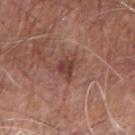Clinical impression:
Imaged during a routine full-body skin examination; the lesion was not biopsied and no histopathology is available.
Clinical summary:
Imaged with white-light lighting. An algorithmic analysis of the crop reported a within-lesion color-variation index near 3/10. A roughly 15 mm field-of-view crop from a total-body skin photograph. Longest diameter approximately 3 mm. The subject is a male roughly 70 years of age. On the right forearm.